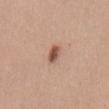No biopsy was performed on this lesion — it was imaged during a full skin examination and was not determined to be concerning. A female subject roughly 50 years of age. The recorded lesion diameter is about 2.5 mm. A close-up tile cropped from a whole-body skin photograph, about 15 mm across. The total-body-photography lesion software estimated a footprint of about 3.5 mm², a shape eccentricity near 0.8, and two-axis asymmetry of about 0.2. The lesion is located on the abdomen. Imaged with white-light lighting.